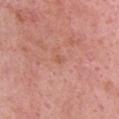No biopsy was performed on this lesion — it was imaged during a full skin examination and was not determined to be concerning. A female subject, approximately 50 years of age. Automated image analysis of the tile measured a lesion area of about 1 mm² and an eccentricity of roughly 0.7. The software also gave an average lesion color of about L≈56 a*≈26 b*≈32 (CIELAB), about 6 CIELAB-L* units darker than the surrounding skin, and a normalized border contrast of about 5. The analysis additionally found a border-irregularity index near 2.5/10 and peripheral color asymmetry of about 0. The software also gave a classifier nevus-likeness of about 0/100 and a detector confidence of about 100 out of 100 that the crop contains a lesion. Cropped from a whole-body photographic skin survey; the tile spans about 15 mm. On the head or neck. Captured under white-light illumination.